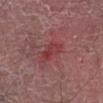  biopsy_status: not biopsied; imaged during a skin examination